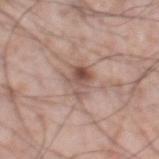Clinical impression:
Imaged during a routine full-body skin examination; the lesion was not biopsied and no histopathology is available.